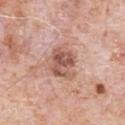biopsy_status: not biopsied; imaged during a skin examination
site: chest
lesion_size:
  long_diameter_mm_approx: 4.0
automated_metrics:
  border_irregularity_0_10: 3.0
  color_variation_0_10: 5.5
lighting: white-light
patient:
  sex: male
  age_approx: 80
image:
  source: total-body photography crop
  field_of_view_mm: 15